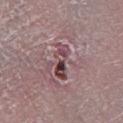Assessment: This lesion was catalogued during total-body skin photography and was not selected for biopsy. Context: From the right lower leg. The lesion's longest dimension is about 4.5 mm. A male patient, roughly 65 years of age. This image is a 15 mm lesion crop taken from a total-body photograph. Imaged with white-light lighting.